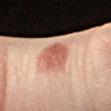workup: no biopsy performed (imaged during a skin exam) | diameter: ≈3.5 mm | tile lighting: cross-polarized illumination | patient: male, roughly 40 years of age | automated metrics: an area of roughly 8 mm², an outline eccentricity of about 0.6 (0 = round, 1 = elongated), and a symmetry-axis asymmetry near 0.2; peripheral color asymmetry of about 1.5; an automated nevus-likeness rating near 20 out of 100 and a detector confidence of about 85 out of 100 that the crop contains a lesion | imaging modality: 15 mm crop, total-body photography | body site: the left forearm.